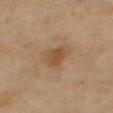Clinical impression:
Captured during whole-body skin photography for melanoma surveillance; the lesion was not biopsied.
Image and clinical context:
From the right thigh. A close-up tile cropped from a whole-body skin photograph, about 15 mm across. The tile uses cross-polarized illumination. A female subject, aged 58–62.